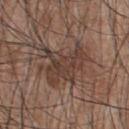Recorded during total-body skin imaging; not selected for excision or biopsy. On the upper back. A male patient aged 43 to 47. Captured under white-light illumination. This image is a 15 mm lesion crop taken from a total-body photograph.